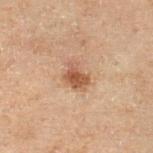Case summary:
– biopsy status: total-body-photography surveillance lesion; no biopsy
– lighting: cross-polarized illumination
– site: the left lower leg
– TBP lesion metrics: an outline eccentricity of about 0.7 (0 = round, 1 = elongated) and a symmetry-axis asymmetry near 0.25; a lesion color around L≈40 a*≈17 b*≈26 in CIELAB, about 10 CIELAB-L* units darker than the surrounding skin, and a normalized border contrast of about 8; an automated nevus-likeness rating near 80 out of 100 and lesion-presence confidence of about 100/100
– subject: male, approximately 70 years of age
– acquisition: ~15 mm tile from a whole-body skin photo